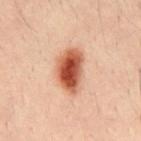biopsy status: catalogued during a skin exam; not biopsied | body site: the chest | subject: male, about 30 years old | lighting: cross-polarized | automated lesion analysis: a lesion area of about 13 mm², an outline eccentricity of about 0.85 (0 = round, 1 = elongated), and a shape-asymmetry score of about 0.25 (0 = symmetric) | imaging modality: ~15 mm tile from a whole-body skin photo | diameter: about 5.5 mm.